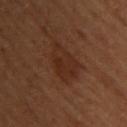The lesion was tiled from a total-body skin photograph and was not biopsied.